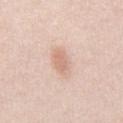Q: Is there a histopathology result?
A: catalogued during a skin exam; not biopsied
Q: Illumination type?
A: white-light
Q: What is the imaging modality?
A: ~15 mm tile from a whole-body skin photo
Q: What is the anatomic site?
A: the chest
Q: What are the patient's age and sex?
A: male, roughly 25 years of age
Q: Automated lesion metrics?
A: a lesion area of about 5.5 mm² and a shape eccentricity near 0.85; a mean CIELAB color near L≈69 a*≈19 b*≈29, about 9 CIELAB-L* units darker than the surrounding skin, and a lesion-to-skin contrast of about 6 (normalized; higher = more distinct)